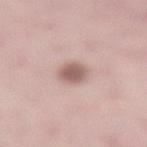Clinical impression:
Part of a total-body skin-imaging series; this lesion was reviewed on a skin check and was not flagged for biopsy.
Context:
Cropped from a total-body skin-imaging series; the visible field is about 15 mm. The lesion's longest dimension is about 3 mm. A female patient, about 50 years old. This is a white-light tile. The lesion is located on the lower back.